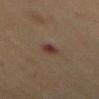<record>
<lighting>cross-polarized</lighting>
<image>
  <source>total-body photography crop</source>
  <field_of_view_mm>15</field_of_view_mm>
</image>
<lesion_size>
  <long_diameter_mm_approx>2.5</long_diameter_mm_approx>
</lesion_size>
<site>mid back</site>
<patient>
  <sex>female</sex>
  <age_approx>70</age_approx>
</patient>
</record>